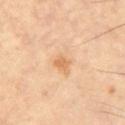Q: Is there a histopathology result?
A: catalogued during a skin exam; not biopsied
Q: What are the patient's age and sex?
A: male, in their 60s
Q: How was the tile lit?
A: cross-polarized
Q: Automated lesion metrics?
A: an average lesion color of about L≈68 a*≈19 b*≈38 (CIELAB), a lesion–skin lightness drop of about 7, and a normalized lesion–skin contrast near 5.5; a border-irregularity rating of about 3.5/10 and radial color variation of about 0.5
Q: What is the imaging modality?
A: ~15 mm crop, total-body skin-cancer survey
Q: Lesion size?
A: about 3.5 mm
Q: What is the anatomic site?
A: the chest